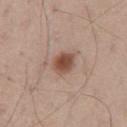Impression:
The lesion was tiled from a total-body skin photograph and was not biopsied.
Image and clinical context:
The lesion is located on the mid back. Imaged with white-light lighting. The subject is a male aged 53 to 57. Automated image analysis of the tile measured border irregularity of about 1.5 on a 0–10 scale and a peripheral color-asymmetry measure near 1. A close-up tile cropped from a whole-body skin photograph, about 15 mm across.